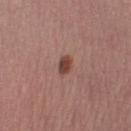The lesion was photographed on a routine skin check and not biopsied; there is no pathology result. Imaged with white-light lighting. A female subject aged 48 to 52. The total-body-photography lesion software estimated a lesion area of about 3.5 mm², a shape eccentricity near 0.75, and a symmetry-axis asymmetry near 0.15. The software also gave a border-irregularity index near 1.5/10, a within-lesion color-variation index near 3/10, and peripheral color asymmetry of about 1. From the leg. A close-up tile cropped from a whole-body skin photograph, about 15 mm across. The recorded lesion diameter is about 2.5 mm.